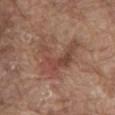Imaged during a routine full-body skin examination; the lesion was not biopsied and no histopathology is available. A 15 mm close-up extracted from a 3D total-body photography capture. About 7 mm across. The lesion-visualizer software estimated an eccentricity of roughly 0.75 and two-axis asymmetry of about 0.6. It also reported a normalized lesion–skin contrast near 6.5. The analysis additionally found a color-variation rating of about 6/10. From the back. Captured under white-light illumination. A male subject, aged approximately 80.